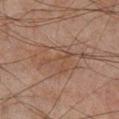The lesion was photographed on a routine skin check and not biopsied; there is no pathology result. The lesion is on the right lower leg. The recorded lesion diameter is about 7.5 mm. The lesion-visualizer software estimated a mean CIELAB color near L≈39 a*≈15 b*≈23 and a normalized lesion–skin contrast near 5. The analysis additionally found a border-irregularity rating of about 6/10, a within-lesion color-variation index near 3.5/10, and a peripheral color-asymmetry measure near 1. A 15 mm crop from a total-body photograph taken for skin-cancer surveillance. The tile uses cross-polarized illumination. A male patient about 45 years old.